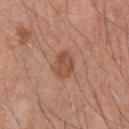Clinical impression: Imaged during a routine full-body skin examination; the lesion was not biopsied and no histopathology is available. Acquisition and patient details: Approximately 3.5 mm at its widest. The tile uses white-light illumination. A 15 mm crop from a total-body photograph taken for skin-cancer surveillance. The subject is a male aged approximately 45. The total-body-photography lesion software estimated a footprint of about 6.5 mm², an eccentricity of roughly 0.7, and a symmetry-axis asymmetry near 0.25. And it measured a lesion color around L≈50 a*≈24 b*≈31 in CIELAB and roughly 9 lightness units darker than nearby skin. The analysis additionally found a border-irregularity rating of about 2.5/10, internal color variation of about 3 on a 0–10 scale, and radial color variation of about 1. It also reported a detector confidence of about 100 out of 100 that the crop contains a lesion. The lesion is on the upper back.